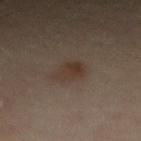<case>
<biopsy_status>not biopsied; imaged during a skin examination</biopsy_status>
<image>
  <source>total-body photography crop</source>
  <field_of_view_mm>15</field_of_view_mm>
</image>
<lesion_size>
  <long_diameter_mm_approx>3.5</long_diameter_mm_approx>
</lesion_size>
<site>left arm</site>
<patient>
  <sex>female</sex>
  <age_approx>30</age_approx>
</patient>
</case>